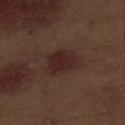| feature | finding |
|---|---|
| lighting | white-light illumination |
| imaging modality | ~15 mm crop, total-body skin-cancer survey |
| anatomic site | the front of the torso |
| lesion size | about 4.5 mm |
| automated metrics | a lesion area of about 10 mm², an outline eccentricity of about 0.75 (0 = round, 1 = elongated), and two-axis asymmetry of about 0.2; an average lesion color of about L≈25 a*≈17 b*≈18 (CIELAB), a lesion–skin lightness drop of about 7, and a normalized lesion–skin contrast near 9; a nevus-likeness score of about 55/100 |
| subject | male, aged 68 to 72 |